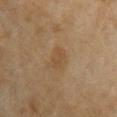Findings:
• notes · catalogued during a skin exam; not biopsied
• lesion size · about 3 mm
• image · total-body-photography crop, ~15 mm field of view
• TBP lesion metrics · a footprint of about 4 mm², a shape eccentricity near 0.85, and two-axis asymmetry of about 0.2; an average lesion color of about L≈44 a*≈15 b*≈31 (CIELAB) and a lesion–skin lightness drop of about 6; a border-irregularity index near 3/10, a within-lesion color-variation index near 1/10, and a peripheral color-asymmetry measure near 0
• site · the arm
• patient · female, about 60 years old